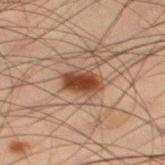A male subject, roughly 55 years of age. A roughly 15 mm field-of-view crop from a total-body skin photograph. Automated tile analysis of the lesion measured an area of roughly 7.5 mm², an eccentricity of roughly 0.7, and a shape-asymmetry score of about 0.2 (0 = symmetric). And it measured a lesion color around L≈36 a*≈19 b*≈27 in CIELAB and a lesion–skin lightness drop of about 13. It also reported internal color variation of about 4 on a 0–10 scale and peripheral color asymmetry of about 1. Captured under cross-polarized illumination. The lesion is located on the left thigh. About 3.5 mm across.